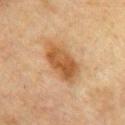Captured during whole-body skin photography for melanoma surveillance; the lesion was not biopsied. The lesion-visualizer software estimated a footprint of about 13 mm², an eccentricity of roughly 0.85, and a symmetry-axis asymmetry near 0.15. The analysis additionally found a lesion color around L≈45 a*≈18 b*≈34 in CIELAB and about 10 CIELAB-L* units darker than the surrounding skin. The software also gave a nevus-likeness score of about 90/100. The lesion is on the chest. A female patient, aged 53–57. Approximately 5.5 mm at its widest. A region of skin cropped from a whole-body photographic capture, roughly 15 mm wide.